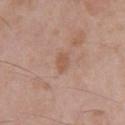Assessment:
Imaged during a routine full-body skin examination; the lesion was not biopsied and no histopathology is available.
Clinical summary:
On the chest. A close-up tile cropped from a whole-body skin photograph, about 15 mm across. A male patient aged 63 to 67. The total-body-photography lesion software estimated an area of roughly 3.5 mm², a shape eccentricity near 0.8, and two-axis asymmetry of about 0.25. It also reported a border-irregularity index near 2.5/10 and internal color variation of about 1.5 on a 0–10 scale. It also reported an automated nevus-likeness rating near 0 out of 100 and a detector confidence of about 100 out of 100 that the crop contains a lesion.